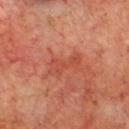This is a cross-polarized tile. A male patient aged 68 to 72. A region of skin cropped from a whole-body photographic capture, roughly 15 mm wide. The recorded lesion diameter is about 4 mm. Located on the chest. The total-body-photography lesion software estimated a footprint of about 6 mm². The analysis additionally found roughly 6 lightness units darker than nearby skin and a normalized lesion–skin contrast near 5. The analysis additionally found an automated nevus-likeness rating near 0 out of 100.